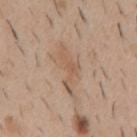location = the back; imaging modality = ~15 mm tile from a whole-body skin photo; TBP lesion metrics = a lesion area of about 6 mm²; patient = male, aged approximately 40; lighting = white-light.